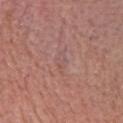Clinical summary:
A close-up tile cropped from a whole-body skin photograph, about 15 mm across. Captured under white-light illumination. The recorded lesion diameter is about 3 mm. The lesion is on the head or neck. A female subject, in their mid- to late 60s. The lesion-visualizer software estimated a shape eccentricity near 0.85 and a symmetry-axis asymmetry near 0.35. It also reported a border-irregularity rating of about 3.5/10 and peripheral color asymmetry of about 1.